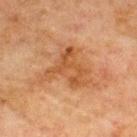Assessment: No biopsy was performed on this lesion — it was imaged during a full skin examination and was not determined to be concerning.